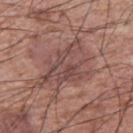Context: A male subject about 55 years old. The lesion is located on the left upper arm. A close-up tile cropped from a whole-body skin photograph, about 15 mm across.